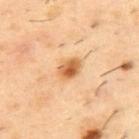site = the upper back | lighting = cross-polarized illumination | lesion size = ~3 mm (longest diameter) | subject = male, roughly 55 years of age | acquisition = total-body-photography crop, ~15 mm field of view.